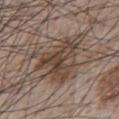The lesion was photographed on a routine skin check and not biopsied; there is no pathology result. The subject is a male approximately 65 years of age. From the chest. A roughly 15 mm field-of-view crop from a total-body skin photograph.